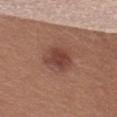A female subject in their mid-50s.
A roughly 15 mm field-of-view crop from a total-body skin photograph.
Longest diameter approximately 3.5 mm.
The lesion is on the left thigh.